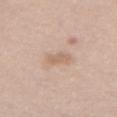Assessment:
No biopsy was performed on this lesion — it was imaged during a full skin examination and was not determined to be concerning.
Background:
Captured under white-light illumination. A female subject aged around 55. From the abdomen. Cropped from a total-body skin-imaging series; the visible field is about 15 mm.